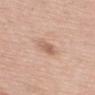No biopsy was performed on this lesion — it was imaged during a full skin examination and was not determined to be concerning. Measured at roughly 2.5 mm in maximum diameter. A female subject about 65 years old. The lesion is located on the chest. The lesion-visualizer software estimated a lesion area of about 4 mm², a shape eccentricity near 0.65, and a symmetry-axis asymmetry near 0.25. The software also gave a mean CIELAB color near L≈61 a*≈20 b*≈30, roughly 9 lightness units darker than nearby skin, and a normalized lesion–skin contrast near 6. And it measured a nevus-likeness score of about 10/100 and a detector confidence of about 100 out of 100 that the crop contains a lesion. A close-up tile cropped from a whole-body skin photograph, about 15 mm across.